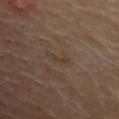  biopsy_status: not biopsied; imaged during a skin examination